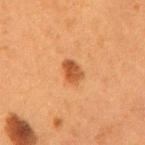Impression:
Captured during whole-body skin photography for melanoma surveillance; the lesion was not biopsied.
Clinical summary:
Located on the mid back. A female subject, aged approximately 50. A region of skin cropped from a whole-body photographic capture, roughly 15 mm wide.